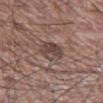The lesion was photographed on a routine skin check and not biopsied; there is no pathology result. This image is a 15 mm lesion crop taken from a total-body photograph. Imaged with white-light lighting. Longest diameter approximately 3.5 mm. From the leg. A male subject roughly 60 years of age.